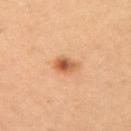follow-up — imaged on a skin check; not biopsied
image source — ~15 mm crop, total-body skin-cancer survey
site — the right upper arm
subject — female, aged 28–32
diameter — ≈2.5 mm